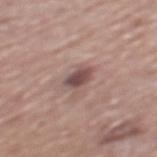Findings:
- notes: catalogued during a skin exam; not biopsied
- lesion size: ≈3 mm
- location: the mid back
- patient: male, roughly 75 years of age
- imaging modality: 15 mm crop, total-body photography